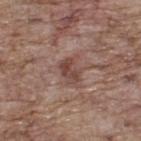Acquisition and patient details: A roughly 15 mm field-of-view crop from a total-body skin photograph. The lesion is located on the upper back. The patient is a male in their 70s. Captured under white-light illumination.